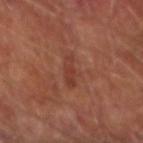  biopsy_status: not biopsied; imaged during a skin examination
  automated_metrics:
    cielab_L: 37
    cielab_a: 24
    cielab_b: 27
    vs_skin_contrast_norm: 5.0
  lesion_size:
    long_diameter_mm_approx: 4.5
  image:
    source: total-body photography crop
    field_of_view_mm: 15
  patient:
    sex: male
    age_approx: 65
  site: right forearm